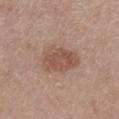Image and clinical context: Automated tile analysis of the lesion measured a lesion area of about 13 mm², an eccentricity of roughly 0.8, and two-axis asymmetry of about 0.25. It also reported about 10 CIELAB-L* units darker than the surrounding skin and a normalized lesion–skin contrast near 7. It also reported border irregularity of about 2.5 on a 0–10 scale and a peripheral color-asymmetry measure near 1. The lesion is located on the left thigh. A lesion tile, about 15 mm wide, cut from a 3D total-body photograph. A male patient aged around 75. Approximately 5 mm at its widest.